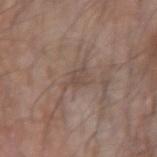<tbp_lesion>
  <biopsy_status>not biopsied; imaged during a skin examination</biopsy_status>
  <lighting>white-light</lighting>
  <patient>
    <sex>male</sex>
    <age_approx>70</age_approx>
  </patient>
  <image>
    <source>total-body photography crop</source>
    <field_of_view_mm>15</field_of_view_mm>
  </image>
  <site>left forearm</site>
</tbp_lesion>